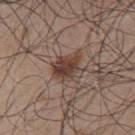<lesion>
  <biopsy_status>not biopsied; imaged during a skin examination</biopsy_status>
  <site>upper back</site>
  <patient>
    <sex>male</sex>
    <age_approx>50</age_approx>
  </patient>
  <image>
    <source>total-body photography crop</source>
    <field_of_view_mm>15</field_of_view_mm>
  </image>
</lesion>